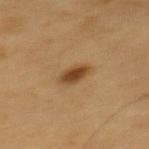image-analysis metrics: an area of roughly 5.5 mm², an eccentricity of roughly 0.85, and a symmetry-axis asymmetry near 0.2; roughly 12 lightness units darker than nearby skin and a normalized border contrast of about 9.5; an automated nevus-likeness rating near 100 out of 100 and lesion-presence confidence of about 100/100 | anatomic site: the mid back | subject: male, approximately 60 years of age | image: 15 mm crop, total-body photography | size: about 3.5 mm | illumination: cross-polarized.